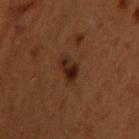Recorded during total-body skin imaging; not selected for excision or biopsy. Captured under cross-polarized illumination. A male patient aged 48 to 52. A 15 mm close-up tile from a total-body photography series done for melanoma screening. On the upper back. Longest diameter approximately 3 mm.